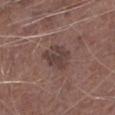This lesion was catalogued during total-body skin photography and was not selected for biopsy. Imaged with white-light lighting. A close-up tile cropped from a whole-body skin photograph, about 15 mm across. The lesion's longest dimension is about 4 mm. On the left lower leg. The subject is a male roughly 75 years of age. Automated tile analysis of the lesion measured a mean CIELAB color near L≈40 a*≈17 b*≈19, roughly 9 lightness units darker than nearby skin, and a lesion-to-skin contrast of about 7.5 (normalized; higher = more distinct). The software also gave border irregularity of about 3 on a 0–10 scale, internal color variation of about 3 on a 0–10 scale, and a peripheral color-asymmetry measure near 1.